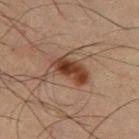The lesion was tiled from a total-body skin photograph and was not biopsied. The recorded lesion diameter is about 5 mm. This is a cross-polarized tile. The lesion is on the left thigh. A male subject, aged 63–67. A 15 mm crop from a total-body photograph taken for skin-cancer surveillance.